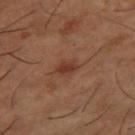Assessment: This lesion was catalogued during total-body skin photography and was not selected for biopsy.